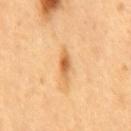A 15 mm close-up extracted from a 3D total-body photography capture. The subject is a male approximately 50 years of age. Located on the mid back.